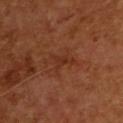{
  "biopsy_status": "not biopsied; imaged during a skin examination",
  "lighting": "cross-polarized",
  "site": "upper back",
  "automated_metrics": {
    "area_mm2_approx": 3.5,
    "eccentricity": 0.75,
    "shape_asymmetry": 0.6
  },
  "image": {
    "source": "total-body photography crop",
    "field_of_view_mm": 15
  },
  "lesion_size": {
    "long_diameter_mm_approx": 3.0
  },
  "patient": {
    "sex": "male",
    "age_approx": 55
  }
}